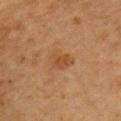* follow-up: imaged on a skin check; not biopsied